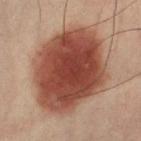The lesion was photographed on a routine skin check and not biopsied; there is no pathology result. The tile uses cross-polarized illumination. A 15 mm close-up tile from a total-body photography series done for melanoma screening. Located on the left leg. The lesion-visualizer software estimated a lesion area of about 65 mm², an outline eccentricity of about 0.65 (0 = round, 1 = elongated), and a symmetry-axis asymmetry near 0.1. And it measured a nevus-likeness score of about 100/100 and a detector confidence of about 100 out of 100 that the crop contains a lesion. The patient is a female aged 38–42. Measured at roughly 10.5 mm in maximum diameter.